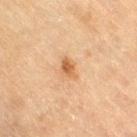workup: total-body-photography surveillance lesion; no biopsy
lighting: cross-polarized
anatomic site: the right thigh
patient: female, aged approximately 80
imaging modality: 15 mm crop, total-body photography
automated lesion analysis: an average lesion color of about L≈53 a*≈20 b*≈36 (CIELAB), about 10 CIELAB-L* units darker than the surrounding skin, and a lesion-to-skin contrast of about 8 (normalized; higher = more distinct); a classifier nevus-likeness of about 65/100 and a lesion-detection confidence of about 100/100
size: ~2.5 mm (longest diameter)